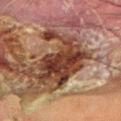{
  "biopsy_status": "not biopsied; imaged during a skin examination",
  "site": "arm",
  "lesion_size": {
    "long_diameter_mm_approx": 8.5
  },
  "patient": {
    "sex": "male",
    "age_approx": 65
  },
  "image": {
    "source": "total-body photography crop",
    "field_of_view_mm": 15
  },
  "lighting": "cross-polarized"
}